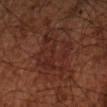No biopsy was performed on this lesion — it was imaged during a full skin examination and was not determined to be concerning.
On the arm.
A male patient about 65 years old.
Imaged with cross-polarized lighting.
Approximately 7.5 mm at its widest.
A 15 mm close-up tile from a total-body photography series done for melanoma screening.